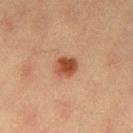Captured during whole-body skin photography for melanoma surveillance; the lesion was not biopsied. A male patient aged approximately 40. Captured under cross-polarized illumination. A roughly 15 mm field-of-view crop from a total-body skin photograph. The lesion is located on the leg. Approximately 2.5 mm at its widest.